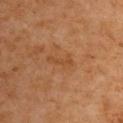Part of a total-body skin-imaging series; this lesion was reviewed on a skin check and was not flagged for biopsy. Approximately 3 mm at its widest. Automated image analysis of the tile measured a lesion area of about 3 mm², an eccentricity of roughly 0.95, and a shape-asymmetry score of about 0.35 (0 = symmetric). The software also gave a nevus-likeness score of about 0/100 and lesion-presence confidence of about 100/100. Cropped from a whole-body photographic skin survey; the tile spans about 15 mm. Located on the upper back. The subject is a male aged 58 to 62. Imaged with cross-polarized lighting.